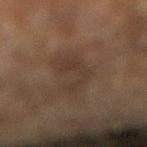follow-up = imaged on a skin check; not biopsied
imaging modality = ~15 mm tile from a whole-body skin photo
size = ≈6 mm
subject = male, about 60 years old
anatomic site = the right lower leg
lighting = cross-polarized